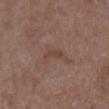Acquisition and patient details:
Imaged with white-light lighting. This image is a 15 mm lesion crop taken from a total-body photograph. The lesion is on the chest. Automated image analysis of the tile measured a lesion-to-skin contrast of about 5.5 (normalized; higher = more distinct). It also reported a border-irregularity index near 3.5/10. And it measured a classifier nevus-likeness of about 0/100 and a detector confidence of about 100 out of 100 that the crop contains a lesion. A female subject, in their 80s.